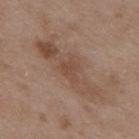Part of a total-body skin-imaging series; this lesion was reviewed on a skin check and was not flagged for biopsy.
Located on the mid back.
Imaged with white-light lighting.
A 15 mm crop from a total-body photograph taken for skin-cancer surveillance.
Longest diameter approximately 3 mm.
The patient is a male aged approximately 50.